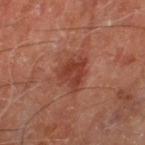Captured during whole-body skin photography for melanoma surveillance; the lesion was not biopsied. The patient is a male approximately 70 years of age. Located on the left thigh. Cropped from a whole-body photographic skin survey; the tile spans about 15 mm. This is a cross-polarized tile.